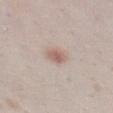Q: Was a biopsy performed?
A: no biopsy performed (imaged during a skin exam)
Q: Illumination type?
A: white-light illumination
Q: Who is the patient?
A: female, about 25 years old
Q: What is the lesion's diameter?
A: about 2.5 mm
Q: Lesion location?
A: the left thigh
Q: How was this image acquired?
A: ~15 mm tile from a whole-body skin photo